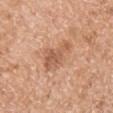This lesion was catalogued during total-body skin photography and was not selected for biopsy.
Captured under white-light illumination.
A female patient roughly 70 years of age.
A close-up tile cropped from a whole-body skin photograph, about 15 mm across.
On the left upper arm.